This image is a 15 mm lesion crop taken from a total-body photograph · a female patient, in their 50s · located on the back.
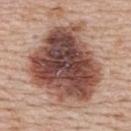Notes:
* lesion size: ≈9.5 mm
* illumination: white-light illumination
* histopathology: a dysplastic (Clark) nevus